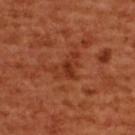Q: Was this lesion biopsied?
A: total-body-photography surveillance lesion; no biopsy
Q: Who is the patient?
A: female, about 55 years old
Q: What kind of image is this?
A: ~15 mm crop, total-body skin-cancer survey
Q: What is the anatomic site?
A: the upper back